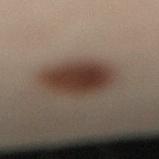Clinical impression: The lesion was photographed on a routine skin check and not biopsied; there is no pathology result. Acquisition and patient details: A male subject aged 48–52. A 15 mm crop from a total-body photograph taken for skin-cancer surveillance. The tile uses cross-polarized illumination. The lesion is located on the left leg.